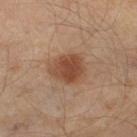Q: Was a biopsy performed?
A: imaged on a skin check; not biopsied
Q: What is the imaging modality?
A: total-body-photography crop, ~15 mm field of view
Q: How was the tile lit?
A: cross-polarized illumination
Q: Where on the body is the lesion?
A: the right thigh
Q: Patient demographics?
A: male, approximately 55 years of age
Q: How large is the lesion?
A: about 4 mm